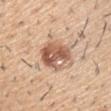The subject is a male aged 58 to 62. A region of skin cropped from a whole-body photographic capture, roughly 15 mm wide. The tile uses white-light illumination. The lesion is on the right upper arm. Approximately 4.5 mm at its widest.